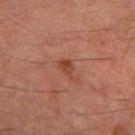{"biopsy_status": "not biopsied; imaged during a skin examination", "image": {"source": "total-body photography crop", "field_of_view_mm": 15}, "lesion_size": {"long_diameter_mm_approx": 2.5}, "site": "leg", "automated_metrics": {"area_mm2_approx": 3.0, "eccentricity": 0.8, "border_irregularity_0_10": 4.0, "color_variation_0_10": 2.0}, "lighting": "cross-polarized", "patient": {"sex": "male", "age_approx": 55}}